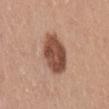Case summary:
– follow-up — catalogued during a skin exam; not biopsied
– subject — female, aged 53 to 57
– image source — total-body-photography crop, ~15 mm field of view
– body site — the mid back